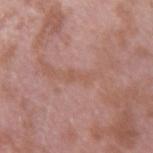The lesion is located on the right upper arm.
The lesion's longest dimension is about 2.5 mm.
A lesion tile, about 15 mm wide, cut from a 3D total-body photograph.
A male patient, aged approximately 40.
Captured under white-light illumination.